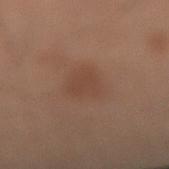Assessment:
Captured during whole-body skin photography for melanoma surveillance; the lesion was not biopsied.
Clinical summary:
A male patient aged 48 to 52. The lesion is on the leg. A close-up tile cropped from a whole-body skin photograph, about 15 mm across. Imaged with cross-polarized lighting.